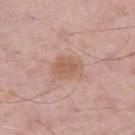About 3.5 mm across.
A close-up tile cropped from a whole-body skin photograph, about 15 mm across.
The total-body-photography lesion software estimated a mean CIELAB color near L≈59 a*≈21 b*≈29, a lesion–skin lightness drop of about 8, and a normalized lesion–skin contrast near 6.5. The analysis additionally found border irregularity of about 2 on a 0–10 scale, internal color variation of about 2 on a 0–10 scale, and peripheral color asymmetry of about 0.5.
On the left thigh.
The subject is a male about 55 years old.
Captured under white-light illumination.